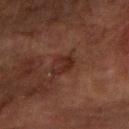Background:
Located on the right forearm. Imaged with cross-polarized lighting. The patient is a male roughly 65 years of age. Approximately 3 mm at its widest. This image is a 15 mm lesion crop taken from a total-body photograph.